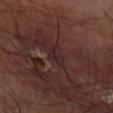Notes:
- biopsy status: total-body-photography surveillance lesion; no biopsy
- image: total-body-photography crop, ~15 mm field of view
- anatomic site: the right forearm
- lighting: cross-polarized
- size: ~3 mm (longest diameter)